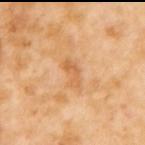Q: Was this lesion biopsied?
A: total-body-photography surveillance lesion; no biopsy
Q: How was this image acquired?
A: ~15 mm tile from a whole-body skin photo
Q: Patient demographics?
A: female, approximately 55 years of age
Q: Where on the body is the lesion?
A: the mid back